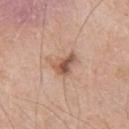location: the left upper arm
lesion diameter: about 3.5 mm
acquisition: ~15 mm crop, total-body skin-cancer survey
tile lighting: white-light
automated metrics: a footprint of about 7 mm²; an average lesion color of about L≈56 a*≈20 b*≈29 (CIELAB), roughly 12 lightness units darker than nearby skin, and a lesion-to-skin contrast of about 8 (normalized; higher = more distinct); a border-irregularity index near 4.5/10, internal color variation of about 7.5 on a 0–10 scale, and radial color variation of about 2.5; a lesion-detection confidence of about 100/100
subject: male, aged approximately 60